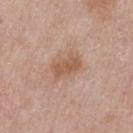notes = catalogued during a skin exam; not biopsied
acquisition = total-body-photography crop, ~15 mm field of view
subject = male, aged 53–57
automated metrics = a lesion area of about 7.5 mm², an eccentricity of roughly 0.8, and two-axis asymmetry of about 0.15; border irregularity of about 2.5 on a 0–10 scale and peripheral color asymmetry of about 0.5
size = ~3.5 mm (longest diameter)
site = the chest